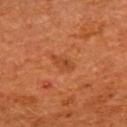<case>
<biopsy_status>not biopsied; imaged during a skin examination</biopsy_status>
<automated_metrics>
  <area_mm2_approx>4.0</area_mm2_approx>
  <eccentricity>0.85</eccentricity>
  <shape_asymmetry>0.3</shape_asymmetry>
</automated_metrics>
<site>upper back</site>
<patient>
  <sex>female</sex>
  <age_approx>65</age_approx>
</patient>
<image>
  <source>total-body photography crop</source>
  <field_of_view_mm>15</field_of_view_mm>
</image>
</case>